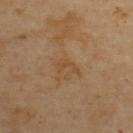Assessment:
This lesion was catalogued during total-body skin photography and was not selected for biopsy.
Background:
An algorithmic analysis of the crop reported a border-irregularity rating of about 7.5/10, a within-lesion color-variation index near 0/10, and radial color variation of about 0. The lesion is on the upper back. About 3 mm across. A lesion tile, about 15 mm wide, cut from a 3D total-body photograph. A male subject aged around 55. Imaged with cross-polarized lighting.